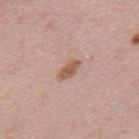| key | value |
|---|---|
| biopsy status | no biopsy performed (imaged during a skin exam) |
| patient | male, aged 43–47 |
| acquisition | ~15 mm crop, total-body skin-cancer survey |
| automated lesion analysis | an area of roughly 3.5 mm², a shape eccentricity near 0.9, and a shape-asymmetry score of about 0.3 (0 = symmetric); an automated nevus-likeness rating near 50 out of 100 and a lesion-detection confidence of about 100/100 |
| lesion size | ≈3 mm |
| illumination | white-light illumination |
| location | the mid back |